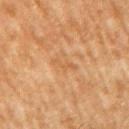Clinical impression: Part of a total-body skin-imaging series; this lesion was reviewed on a skin check and was not flagged for biopsy. Context: From the right upper arm. Cropped from a total-body skin-imaging series; the visible field is about 15 mm. The recorded lesion diameter is about 3.5 mm. Imaged with cross-polarized lighting. A male patient in their mid-60s. Automated image analysis of the tile measured roughly 6 lightness units darker than nearby skin and a normalized lesion–skin contrast near 4.5.